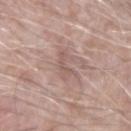Impression: No biopsy was performed on this lesion — it was imaged during a full skin examination and was not determined to be concerning. Acquisition and patient details: A 15 mm close-up extracted from a 3D total-body photography capture. The lesion is located on the left forearm. Captured under white-light illumination. A male patient aged approximately 75. Approximately 3 mm at its widest.